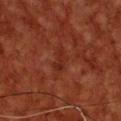<record>
<biopsy_status>not biopsied; imaged during a skin examination</biopsy_status>
<patient>
  <sex>male</sex>
  <age_approx>60</age_approx>
</patient>
<automated_metrics>
  <area_mm2_approx>5.0</area_mm2_approx>
  <eccentricity>0.85</eccentricity>
  <shape_asymmetry>0.4</shape_asymmetry>
  <cielab_L>24</cielab_L>
  <cielab_a>24</cielab_a>
  <cielab_b>26</cielab_b>
  <vs_skin_darker_L>4.0</vs_skin_darker_L>
  <vs_skin_contrast_norm>4.5</vs_skin_contrast_norm>
  <border_irregularity_0_10>4.0</border_irregularity_0_10>
  <color_variation_0_10>4.0</color_variation_0_10>
  <peripheral_color_asymmetry>1.5</peripheral_color_asymmetry>
  <lesion_detection_confidence_0_100>100</lesion_detection_confidence_0_100>
</automated_metrics>
<image>
  <source>total-body photography crop</source>
  <field_of_view_mm>15</field_of_view_mm>
</image>
<lighting>cross-polarized</lighting>
<site>chest</site>
</record>